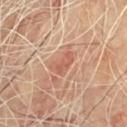Impression: Part of a total-body skin-imaging series; this lesion was reviewed on a skin check and was not flagged for biopsy. Context: Approximately 3.5 mm at its widest. Captured under cross-polarized illumination. Automated tile analysis of the lesion measured a mean CIELAB color near L≈57 a*≈25 b*≈31 and roughly 8 lightness units darker than nearby skin. The analysis additionally found a within-lesion color-variation index near 2/10 and radial color variation of about 0.5. And it measured an automated nevus-likeness rating near 85 out of 100 and lesion-presence confidence of about 100/100. A region of skin cropped from a whole-body photographic capture, roughly 15 mm wide. The lesion is located on the chest. The subject is a male approximately 70 years of age.